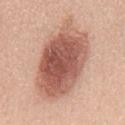Q: Lesion size?
A: about 10.5 mm
Q: What kind of image is this?
A: total-body-photography crop, ~15 mm field of view
Q: Lesion location?
A: the mid back
Q: What lighting was used for the tile?
A: white-light illumination
Q: What are the patient's age and sex?
A: female, about 40 years old